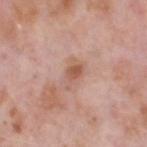This lesion was catalogued during total-body skin photography and was not selected for biopsy.
A region of skin cropped from a whole-body photographic capture, roughly 15 mm wide.
A male patient aged 68–72.
Located on the head or neck.
An algorithmic analysis of the crop reported about 9 CIELAB-L* units darker than the surrounding skin. It also reported border irregularity of about 4 on a 0–10 scale, a color-variation rating of about 3.5/10, and a peripheral color-asymmetry measure near 1.
Imaged with white-light lighting.